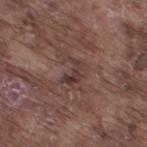This lesion was catalogued during total-body skin photography and was not selected for biopsy. A close-up tile cropped from a whole-body skin photograph, about 15 mm across. Automated image analysis of the tile measured internal color variation of about 0 on a 0–10 scale and a peripheral color-asymmetry measure near 0. It also reported an automated nevus-likeness rating near 0 out of 100 and a lesion-detection confidence of about 95/100. A male patient, aged approximately 75. On the right thigh.